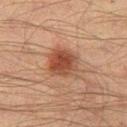Findings:
- workup · total-body-photography surveillance lesion; no biopsy
- patient · male, in their mid-40s
- image source · total-body-photography crop, ~15 mm field of view
- diameter · ~4 mm (longest diameter)
- anatomic site · the leg
- lighting · cross-polarized